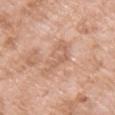<case>
<patient>
  <sex>female</sex>
  <age_approx>75</age_approx>
</patient>
<lighting>white-light</lighting>
<lesion_size>
  <long_diameter_mm_approx>4.5</long_diameter_mm_approx>
</lesion_size>
<site>arm</site>
<image>
  <source>total-body photography crop</source>
  <field_of_view_mm>15</field_of_view_mm>
</image>
</case>